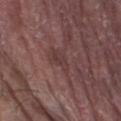- notes — imaged on a skin check; not biopsied
- body site — the left thigh
- lighting — white-light
- subject — male, in their 80s
- image — 15 mm crop, total-body photography
- diameter — ~3 mm (longest diameter)